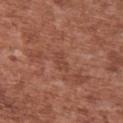• subject: male, approximately 45 years of age
• image source: ~15 mm crop, total-body skin-cancer survey
• anatomic site: the chest
• lighting: white-light illumination